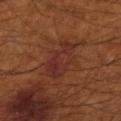Impression: Imaged during a routine full-body skin examination; the lesion was not biopsied and no histopathology is available. Image and clinical context: On the right forearm. A male subject aged approximately 55. A roughly 15 mm field-of-view crop from a total-body skin photograph.